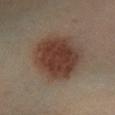– notes · no biopsy performed (imaged during a skin exam)
– image source · ~15 mm crop, total-body skin-cancer survey
– subject · male, in their 40s
– body site · the leg